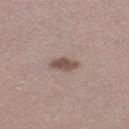Assessment: The lesion was tiled from a total-body skin photograph and was not biopsied. Clinical summary: A region of skin cropped from a whole-body photographic capture, roughly 15 mm wide. Imaged with white-light lighting. The lesion's longest dimension is about 3.5 mm. Automated image analysis of the tile measured a footprint of about 5 mm², a shape eccentricity near 0.8, and a symmetry-axis asymmetry near 0.2. And it measured a classifier nevus-likeness of about 90/100. A female patient, in their mid-20s. The lesion is on the left thigh.